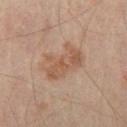site: right thigh
lesion_size:
  long_diameter_mm_approx: 5.5
patient:
  age_approx: 55
image:
  source: total-body photography crop
  field_of_view_mm: 15